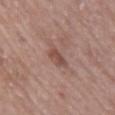Clinical impression: No biopsy was performed on this lesion — it was imaged during a full skin examination and was not determined to be concerning. Context: Imaged with white-light lighting. Cropped from a total-body skin-imaging series; the visible field is about 15 mm. A female patient, in their mid- to late 60s. The lesion's longest dimension is about 2.5 mm. The total-body-photography lesion software estimated a lesion area of about 3.5 mm² and a symmetry-axis asymmetry near 0.25. The software also gave border irregularity of about 3 on a 0–10 scale and a peripheral color-asymmetry measure near 0.5. On the right upper arm.